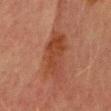The lesion was photographed on a routine skin check and not biopsied; there is no pathology result. The recorded lesion diameter is about 8.5 mm. The subject is a male approximately 70 years of age. Automated tile analysis of the lesion measured a border-irregularity index near 5/10, a within-lesion color-variation index near 4/10, and peripheral color asymmetry of about 1.5. A lesion tile, about 15 mm wide, cut from a 3D total-body photograph. The lesion is located on the head or neck.